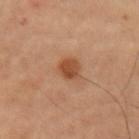workup: no biopsy performed (imaged during a skin exam); subject: male, in their mid- to late 50s; lighting: cross-polarized; location: the left upper arm; image: ~15 mm crop, total-body skin-cancer survey; lesion diameter: about 2.5 mm.